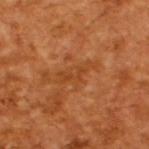{
  "biopsy_status": "not biopsied; imaged during a skin examination",
  "lesion_size": {
    "long_diameter_mm_approx": 4.5
  },
  "image": {
    "source": "total-body photography crop",
    "field_of_view_mm": 15
  },
  "patient": {
    "sex": "male",
    "age_approx": 65
  },
  "lighting": "cross-polarized",
  "automated_metrics": {
    "border_irregularity_0_10": 8.5,
    "nevus_likeness_0_100": 0,
    "lesion_detection_confidence_0_100": 100
  }
}